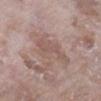{"biopsy_status": "not biopsied; imaged during a skin examination", "image": {"source": "total-body photography crop", "field_of_view_mm": 15}, "lighting": "white-light", "lesion_size": {"long_diameter_mm_approx": 5.5}, "automated_metrics": {"area_mm2_approx": 10.0, "shape_asymmetry": 0.45, "nevus_likeness_0_100": 0, "lesion_detection_confidence_0_100": 80}, "site": "left lower leg", "patient": {"sex": "female", "age_approx": 70}}